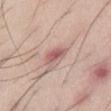follow-up: imaged on a skin check; not biopsied
body site: the abdomen
subject: male, aged 33–37
imaging modality: ~15 mm tile from a whole-body skin photo